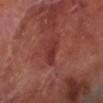follow-up: catalogued during a skin exam; not biopsied
TBP lesion metrics: an area of roughly 6 mm², a shape eccentricity near 0.85, and a shape-asymmetry score of about 0.3 (0 = symmetric); a nevus-likeness score of about 0/100 and a lesion-detection confidence of about 85/100
tile lighting: cross-polarized
anatomic site: the left lower leg
size: ≈3.5 mm
imaging modality: ~15 mm crop, total-body skin-cancer survey
subject: male, aged approximately 70